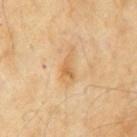Q: Was a biopsy performed?
A: total-body-photography surveillance lesion; no biopsy
Q: How large is the lesion?
A: about 4 mm
Q: How was this image acquired?
A: total-body-photography crop, ~15 mm field of view
Q: How was the tile lit?
A: cross-polarized illumination
Q: Patient demographics?
A: male, about 60 years old
Q: Where on the body is the lesion?
A: the abdomen